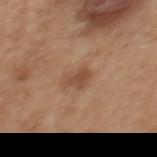| key | value |
|---|---|
| workup | total-body-photography surveillance lesion; no biopsy |
| diameter | ≈2.5 mm |
| location | the upper back |
| subject | female, aged around 40 |
| imaging modality | 15 mm crop, total-body photography |
| tile lighting | white-light |